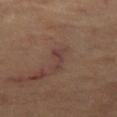The lesion was photographed on a routine skin check and not biopsied; there is no pathology result. The subject is approximately 60 years of age. The total-body-photography lesion software estimated a border-irregularity index near 5/10. The lesion's longest dimension is about 3 mm. Located on the left thigh. A 15 mm close-up tile from a total-body photography series done for melanoma screening.